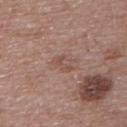The lesion was photographed on a routine skin check and not biopsied; there is no pathology result. The lesion is located on the upper back. A 15 mm crop from a total-body photograph taken for skin-cancer surveillance. Imaged with white-light lighting. The lesion-visualizer software estimated a lesion area of about 3 mm² and two-axis asymmetry of about 0.45. The software also gave a nevus-likeness score of about 0/100 and lesion-presence confidence of about 100/100. The patient is a female aged 63–67.